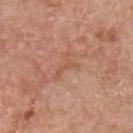site: chest
lighting: white-light
lesion_size:
  long_diameter_mm_approx: 3.5
image:
  source: total-body photography crop
  field_of_view_mm: 15
patient:
  sex: male
  age_approx: 60